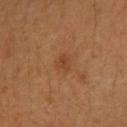Clinical impression:
This lesion was catalogued during total-body skin photography and was not selected for biopsy.
Acquisition and patient details:
The patient is a female aged 53 to 57. Located on the left forearm. Approximately 2 mm at its widest. Cropped from a total-body skin-imaging series; the visible field is about 15 mm. The lesion-visualizer software estimated an outline eccentricity of about 0.5 (0 = round, 1 = elongated) and a shape-asymmetry score of about 0.45 (0 = symmetric). It also reported a border-irregularity index near 3.5/10, a color-variation rating of about 0.5/10, and radial color variation of about 0.